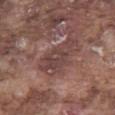follow-up = no biopsy performed (imaged during a skin exam) | anatomic site = the left thigh | image source = 15 mm crop, total-body photography | automated lesion analysis = a footprint of about 14 mm² | lighting = white-light | size = about 6 mm | patient = male, aged 73 to 77.